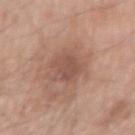This lesion was catalogued during total-body skin photography and was not selected for biopsy. A male patient, in their mid- to late 50s. The lesion is located on the right upper arm. Cropped from a whole-body photographic skin survey; the tile spans about 15 mm.